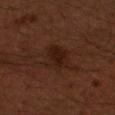Assessment:
This lesion was catalogued during total-body skin photography and was not selected for biopsy.
Acquisition and patient details:
An algorithmic analysis of the crop reported a lesion area of about 8.5 mm², a shape eccentricity near 0.45, and two-axis asymmetry of about 0.25. And it measured a mean CIELAB color near L≈17 a*≈17 b*≈20, roughly 5 lightness units darker than nearby skin, and a normalized border contrast of about 7. The software also gave border irregularity of about 2.5 on a 0–10 scale and a peripheral color-asymmetry measure near 1. The software also gave an automated nevus-likeness rating near 65 out of 100 and lesion-presence confidence of about 100/100. The lesion is located on the arm. A male subject, aged 58–62. A close-up tile cropped from a whole-body skin photograph, about 15 mm across.